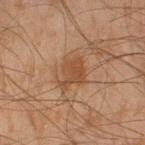This is a cross-polarized tile.
A lesion tile, about 15 mm wide, cut from a 3D total-body photograph.
The subject is a male roughly 45 years of age.
An algorithmic analysis of the crop reported a color-variation rating of about 1.5/10 and peripheral color asymmetry of about 0.5.
The recorded lesion diameter is about 4 mm.
The lesion is located on the right thigh.